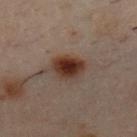lesion diameter: ≈3.5 mm
image-analysis metrics: an outline eccentricity of about 0.25 (0 = round, 1 = elongated) and a symmetry-axis asymmetry near 0.15; a mean CIELAB color near L≈27 a*≈15 b*≈20 and a normalized border contrast of about 11.5
image source: ~15 mm crop, total-body skin-cancer survey
location: the chest
subject: male, aged 28–32
tile lighting: cross-polarized illumination